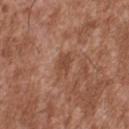Clinical impression: This lesion was catalogued during total-body skin photography and was not selected for biopsy. Context: Measured at roughly 3.5 mm in maximum diameter. Located on the upper back. A region of skin cropped from a whole-body photographic capture, roughly 15 mm wide. A male patient in their mid- to late 40s.